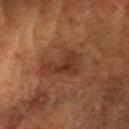Q: What did automated image analysis measure?
A: a shape eccentricity near 0.85; roughly 6 lightness units darker than nearby skin; a classifier nevus-likeness of about 5/100 and a lesion-detection confidence of about 100/100
Q: Who is the patient?
A: female, aged 78 to 82
Q: What is the lesion's diameter?
A: ~5 mm (longest diameter)
Q: Lesion location?
A: the head or neck
Q: How was this image acquired?
A: ~15 mm crop, total-body skin-cancer survey
Q: Illumination type?
A: cross-polarized illumination